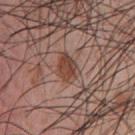{"biopsy_status": "not biopsied; imaged during a skin examination", "lighting": "white-light", "patient": {"sex": "male", "age_approx": 55}, "image": {"source": "total-body photography crop", "field_of_view_mm": 15}, "site": "chest"}